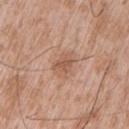{"biopsy_status": "not biopsied; imaged during a skin examination", "image": {"source": "total-body photography crop", "field_of_view_mm": 15}, "patient": {"sex": "male", "age_approx": 50}, "lesion_size": {"long_diameter_mm_approx": 2.5}, "site": "left upper arm", "lighting": "white-light"}